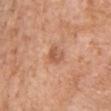This lesion was catalogued during total-body skin photography and was not selected for biopsy. Measured at roughly 2.5 mm in maximum diameter. The lesion-visualizer software estimated a lesion area of about 4 mm² and an eccentricity of roughly 0.7. It also reported an average lesion color of about L≈57 a*≈24 b*≈34 (CIELAB), about 10 CIELAB-L* units darker than the surrounding skin, and a lesion-to-skin contrast of about 6.5 (normalized; higher = more distinct). The analysis additionally found an automated nevus-likeness rating near 0 out of 100 and lesion-presence confidence of about 100/100. On the chest. The tile uses white-light illumination. The subject is a male roughly 60 years of age. A roughly 15 mm field-of-view crop from a total-body skin photograph.